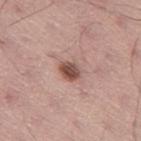Impression: The lesion was photographed on a routine skin check and not biopsied; there is no pathology result. Context: Longest diameter approximately 3 mm. On the left thigh. A male subject, aged approximately 55. A 15 mm close-up tile from a total-body photography series done for melanoma screening. The tile uses white-light illumination. An algorithmic analysis of the crop reported a shape eccentricity near 0.65 and a symmetry-axis asymmetry near 0.2. The analysis additionally found border irregularity of about 1.5 on a 0–10 scale, internal color variation of about 4 on a 0–10 scale, and a peripheral color-asymmetry measure near 1. It also reported an automated nevus-likeness rating near 90 out of 100 and lesion-presence confidence of about 100/100.